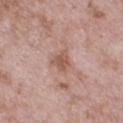The lesion was photographed on a routine skin check and not biopsied; there is no pathology result. The lesion's longest dimension is about 3 mm. A 15 mm crop from a total-body photograph taken for skin-cancer surveillance. The lesion-visualizer software estimated a color-variation rating of about 2/10 and a peripheral color-asymmetry measure near 0.5. And it measured a lesion-detection confidence of about 100/100. On the chest. A male subject, aged approximately 50. The tile uses white-light illumination.